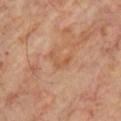* workup — no biopsy performed (imaged during a skin exam)
* patient — male, roughly 70 years of age
* TBP lesion metrics — a border-irregularity rating of about 7.5/10, a within-lesion color-variation index near 0/10, and peripheral color asymmetry of about 0
* diameter — about 3 mm
* image source — ~15 mm tile from a whole-body skin photo
* location — the chest